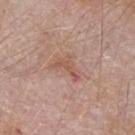Q: How large is the lesion?
A: ≈3.5 mm
Q: How was the tile lit?
A: white-light
Q: Patient demographics?
A: male, aged 63 to 67
Q: What is the anatomic site?
A: the left forearm
Q: What kind of image is this?
A: total-body-photography crop, ~15 mm field of view